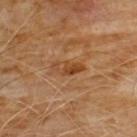The lesion was tiled from a total-body skin photograph and was not biopsied. The tile uses cross-polarized illumination. Longest diameter approximately 3.5 mm. Cropped from a whole-body photographic skin survey; the tile spans about 15 mm. The subject is a male in their 60s. The lesion is located on the front of the torso.